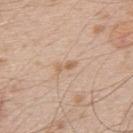<record>
<biopsy_status>not biopsied; imaged during a skin examination</biopsy_status>
<patient>
  <sex>male</sex>
  <age_approx>50</age_approx>
</patient>
<lighting>white-light</lighting>
<image>
  <source>total-body photography crop</source>
  <field_of_view_mm>15</field_of_view_mm>
</image>
<lesion_size>
  <long_diameter_mm_approx>2.5</long_diameter_mm_approx>
</lesion_size>
<site>upper back</site>
</record>